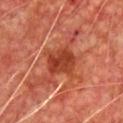follow-up: total-body-photography surveillance lesion; no biopsy
illumination: cross-polarized
anatomic site: the front of the torso
acquisition: ~15 mm tile from a whole-body skin photo
automated lesion analysis: an area of roughly 9.5 mm², a shape eccentricity near 0.55, and two-axis asymmetry of about 0.25; an average lesion color of about L≈37 a*≈30 b*≈33 (CIELAB), about 10 CIELAB-L* units darker than the surrounding skin, and a lesion-to-skin contrast of about 8 (normalized; higher = more distinct); a border-irregularity rating of about 2.5/10, a within-lesion color-variation index near 3.5/10, and radial color variation of about 1; a detector confidence of about 100 out of 100 that the crop contains a lesion
patient: male, in their mid-60s